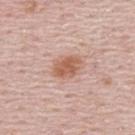Notes:
• follow-up · no biopsy performed (imaged during a skin exam)
• acquisition · ~15 mm tile from a whole-body skin photo
• patient · male, approximately 50 years of age
• anatomic site · the back
• illumination · white-light